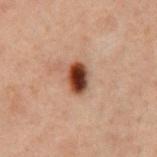Clinical impression:
Recorded during total-body skin imaging; not selected for excision or biopsy.
Image and clinical context:
Imaged with cross-polarized lighting. A male subject, aged approximately 60. The lesion is located on the chest. Automated tile analysis of the lesion measured an average lesion color of about L≈32 a*≈19 b*≈25 (CIELAB) and about 17 CIELAB-L* units darker than the surrounding skin. And it measured a border-irregularity rating of about 1.5/10, a within-lesion color-variation index near 7.5/10, and radial color variation of about 2.5. The lesion's longest dimension is about 3.5 mm. This image is a 15 mm lesion crop taken from a total-body photograph.